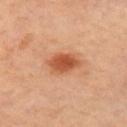biopsy status: total-body-photography surveillance lesion; no biopsy
diameter: about 4 mm
subject: female, aged 63 to 67
tile lighting: cross-polarized
automated metrics: a nevus-likeness score of about 100/100 and a detector confidence of about 100 out of 100 that the crop contains a lesion
body site: the right upper arm
imaging modality: total-body-photography crop, ~15 mm field of view